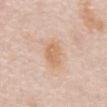Q: Was a biopsy performed?
A: total-body-photography surveillance lesion; no biopsy
Q: What is the imaging modality?
A: total-body-photography crop, ~15 mm field of view
Q: How large is the lesion?
A: ≈4 mm
Q: How was the tile lit?
A: white-light
Q: What are the patient's age and sex?
A: male, in their mid-70s
Q: What did automated image analysis measure?
A: a shape eccentricity near 0.7; a lesion–skin lightness drop of about 8 and a normalized border contrast of about 6.5; border irregularity of about 2 on a 0–10 scale and a color-variation rating of about 3/10; a detector confidence of about 100 out of 100 that the crop contains a lesion
Q: Where on the body is the lesion?
A: the abdomen